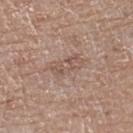| field | value |
|---|---|
| workup | no biopsy performed (imaged during a skin exam) |
| image-analysis metrics | a lesion area of about 8 mm², an eccentricity of roughly 0.85, and a symmetry-axis asymmetry near 0.3; a border-irregularity rating of about 3.5/10, internal color variation of about 3.5 on a 0–10 scale, and a peripheral color-asymmetry measure near 1; a classifier nevus-likeness of about 0/100 and a detector confidence of about 95 out of 100 that the crop contains a lesion |
| site | the right lower leg |
| patient | female, aged approximately 75 |
| diameter | ~4.5 mm (longest diameter) |
| imaging modality | total-body-photography crop, ~15 mm field of view |
| lighting | white-light |